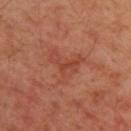Part of a total-body skin-imaging series; this lesion was reviewed on a skin check and was not flagged for biopsy.